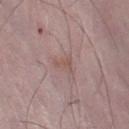biopsy_status: not biopsied; imaged during a skin examination
site: right lower leg
patient:
  sex: male
  age_approx: 50
image:
  source: total-body photography crop
  field_of_view_mm: 15